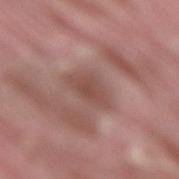Assessment:
No biopsy was performed on this lesion — it was imaged during a full skin examination and was not determined to be concerning.
Image and clinical context:
Longest diameter approximately 4 mm. Automated image analysis of the tile measured an outline eccentricity of about 0.7 (0 = round, 1 = elongated). It also reported a lesion color around L≈49 a*≈20 b*≈23 in CIELAB, a lesion–skin lightness drop of about 8, and a normalized lesion–skin contrast near 6.5. The software also gave a border-irregularity index near 2.5/10, a within-lesion color-variation index near 2.5/10, and peripheral color asymmetry of about 0.5. The software also gave a nevus-likeness score of about 0/100 and a lesion-detection confidence of about 100/100. A male patient roughly 40 years of age. Located on the lower back. Imaged with white-light lighting. Cropped from a whole-body photographic skin survey; the tile spans about 15 mm.